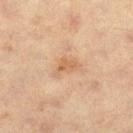  site: left thigh
  automated_metrics:
    area_mm2_approx: 3.5
    eccentricity: 0.75
    shape_asymmetry: 0.45
    border_irregularity_0_10: 4.0
    peripheral_color_asymmetry: 0.5
    nevus_likeness_0_100: 0
  patient:
    sex: male
    age_approx: 60
  lighting: cross-polarized
  image:
    source: total-body photography crop
    field_of_view_mm: 15